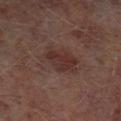Clinical impression:
Imaged during a routine full-body skin examination; the lesion was not biopsied and no histopathology is available.
Image and clinical context:
Captured under cross-polarized illumination. The total-body-photography lesion software estimated an area of roughly 11 mm² and an outline eccentricity of about 0.75 (0 = round, 1 = elongated). And it measured a lesion color around L≈30 a*≈18 b*≈20 in CIELAB, about 7 CIELAB-L* units darker than the surrounding skin, and a normalized border contrast of about 7. The software also gave a border-irregularity index near 3/10 and a within-lesion color-variation index near 4/10. The subject is a male roughly 65 years of age. Approximately 4.5 mm at its widest. Located on the left lower leg. Cropped from a whole-body photographic skin survey; the tile spans about 15 mm.